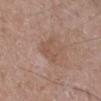The lesion was tiled from a total-body skin photograph and was not biopsied.
A male patient, aged 48 to 52.
Captured under white-light illumination.
The lesion is located on the right lower leg.
A lesion tile, about 15 mm wide, cut from a 3D total-body photograph.